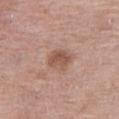Q: Was a biopsy performed?
A: total-body-photography surveillance lesion; no biopsy
Q: Lesion location?
A: the right thigh
Q: Patient demographics?
A: female, in their mid- to late 60s
Q: What is the imaging modality?
A: ~15 mm crop, total-body skin-cancer survey